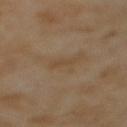Q: What lighting was used for the tile?
A: cross-polarized
Q: What is the lesion's diameter?
A: ~2.5 mm (longest diameter)
Q: Patient demographics?
A: female, in their mid-50s
Q: What is the anatomic site?
A: the back
Q: What is the imaging modality?
A: ~15 mm crop, total-body skin-cancer survey